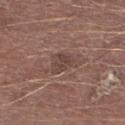Q: Was this lesion biopsied?
A: no biopsy performed (imaged during a skin exam)
Q: What is the imaging modality?
A: ~15 mm crop, total-body skin-cancer survey
Q: How was the tile lit?
A: white-light illumination
Q: Where on the body is the lesion?
A: the leg
Q: What are the patient's age and sex?
A: male, in their mid-70s
Q: What is the lesion's diameter?
A: ≈3 mm
Q: What did automated image analysis measure?
A: a footprint of about 5 mm² and an outline eccentricity of about 0.7 (0 = round, 1 = elongated); a border-irregularity index near 3.5/10 and internal color variation of about 2 on a 0–10 scale